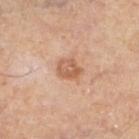Recorded during total-body skin imaging; not selected for excision or biopsy. A 15 mm close-up extracted from a 3D total-body photography capture. The tile uses cross-polarized illumination. The lesion is located on the left lower leg. A male patient aged 63 to 67. The recorded lesion diameter is about 3 mm.